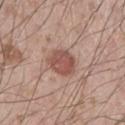Imaged during a routine full-body skin examination; the lesion was not biopsied and no histopathology is available. This is a white-light tile. The patient is a male in their mid- to late 30s. A 15 mm close-up extracted from a 3D total-body photography capture. Automated image analysis of the tile measured a lesion area of about 9 mm², an outline eccentricity of about 0.65 (0 = round, 1 = elongated), and two-axis asymmetry of about 0.15. And it measured roughly 11 lightness units darker than nearby skin and a lesion-to-skin contrast of about 8 (normalized; higher = more distinct). The analysis additionally found an automated nevus-likeness rating near 80 out of 100 and lesion-presence confidence of about 100/100. About 4 mm across. On the left lower leg.